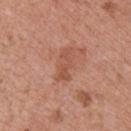Imaged during a routine full-body skin examination; the lesion was not biopsied and no histopathology is available.